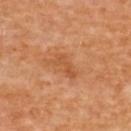Recorded during total-body skin imaging; not selected for excision or biopsy. The lesion is located on the upper back. A 15 mm close-up tile from a total-body photography series done for melanoma screening. The tile uses cross-polarized illumination. A female patient, aged 48–52.